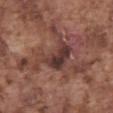This lesion was catalogued during total-body skin photography and was not selected for biopsy.